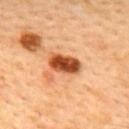Background:
Imaged with cross-polarized lighting. The lesion is located on the upper back. The recorded lesion diameter is about 4 mm. A male patient aged around 45. Cropped from a whole-body photographic skin survey; the tile spans about 15 mm.